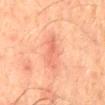Assessment: Captured during whole-body skin photography for melanoma surveillance; the lesion was not biopsied. Background: Captured under cross-polarized illumination. A 15 mm crop from a total-body photograph taken for skin-cancer surveillance. A male patient, in their mid- to late 60s. The lesion is on the back. About 4.5 mm across.